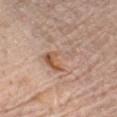  biopsy_status: not biopsied; imaged during a skin examination
  image:
    source: total-body photography crop
    field_of_view_mm: 15
  site: chest
  lighting: white-light
  lesion_size:
    long_diameter_mm_approx: 5.0
  patient:
    sex: male
    age_approx: 70